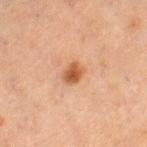This is a cross-polarized tile. The lesion is on the leg. Automated image analysis of the tile measured a lesion area of about 4 mm² and a symmetry-axis asymmetry near 0.25. The software also gave a classifier nevus-likeness of about 95/100 and a detector confidence of about 100 out of 100 that the crop contains a lesion. A lesion tile, about 15 mm wide, cut from a 3D total-body photograph. A female subject aged approximately 55. About 2.5 mm across.